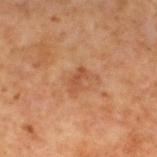The lesion was tiled from a total-body skin photograph and was not biopsied.
The lesion is located on the left lower leg.
Approximately 2.5 mm at its widest.
The patient is a male approximately 60 years of age.
A region of skin cropped from a whole-body photographic capture, roughly 15 mm wide.
The tile uses cross-polarized illumination.